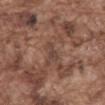follow-up — imaged on a skin check; not biopsied | subject — male, aged 73 to 77 | body site — the abdomen | automated metrics — an average lesion color of about L≈42 a*≈18 b*≈23 (CIELAB) and a normalized lesion–skin contrast near 5.5; border irregularity of about 4.5 on a 0–10 scale, a within-lesion color-variation index near 0.5/10, and peripheral color asymmetry of about 0; a nevus-likeness score of about 0/100 and lesion-presence confidence of about 75/100 | image — ~15 mm crop, total-body skin-cancer survey | illumination — white-light illumination | lesion diameter — ~3 mm (longest diameter).